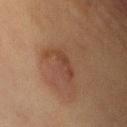| field | value |
|---|---|
| workup | total-body-photography surveillance lesion; no biopsy |
| subject | female, aged 58–62 |
| imaging modality | total-body-photography crop, ~15 mm field of view |
| illumination | cross-polarized illumination |
| diameter | about 5 mm |
| automated metrics | a border-irregularity rating of about 7.5/10, a color-variation rating of about 1/10, and a peripheral color-asymmetry measure near 0.5 |
| anatomic site | the front of the torso |